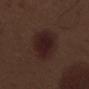biopsy_status: not biopsied; imaged during a skin examination
image:
  source: total-body photography crop
  field_of_view_mm: 15
patient:
  sex: male
  age_approx: 70
lesion_size:
  long_diameter_mm_approx: 5.0
lighting: white-light
site: left thigh